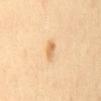Impression: Part of a total-body skin-imaging series; this lesion was reviewed on a skin check and was not flagged for biopsy. Image and clinical context: Captured under cross-polarized illumination. The patient is a female about 60 years old. The lesion is on the mid back. Automated tile analysis of the lesion measured a border-irregularity index near 2/10, internal color variation of about 3.5 on a 0–10 scale, and a peripheral color-asymmetry measure near 1.5. The software also gave a classifier nevus-likeness of about 90/100. A region of skin cropped from a whole-body photographic capture, roughly 15 mm wide.